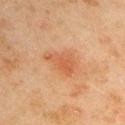{"image": {"source": "total-body photography crop", "field_of_view_mm": 15}, "site": "right upper arm", "patient": {"sex": "male", "age_approx": 45}, "lesion_size": {"long_diameter_mm_approx": 4.0}, "automated_metrics": {"vs_skin_darker_L": 7.0}}